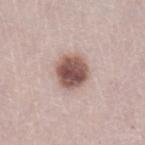No biopsy was performed on this lesion — it was imaged during a full skin examination and was not determined to be concerning. A female subject, aged 48–52. Located on the left lower leg. A close-up tile cropped from a whole-body skin photograph, about 15 mm across. About 4 mm across.